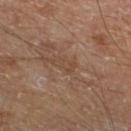notes — imaged on a skin check; not biopsied | location — the left lower leg | patient — aged approximately 55 | illumination — cross-polarized | image source — 15 mm crop, total-body photography | size — ~3 mm (longest diameter) | automated metrics — a footprint of about 3.5 mm², a shape eccentricity near 0.85, and a symmetry-axis asymmetry near 0.4; a border-irregularity index near 4.5/10 and a within-lesion color-variation index near 1/10; a nevus-likeness score of about 0/100 and a lesion-detection confidence of about 90/100.